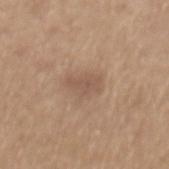follow-up = imaged on a skin check; not biopsied
anatomic site = the mid back
image source = ~15 mm tile from a whole-body skin photo
subject = male, aged 63 to 67